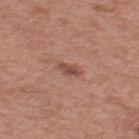The lesion was tiled from a total-body skin photograph and was not biopsied.
The tile uses white-light illumination.
A region of skin cropped from a whole-body photographic capture, roughly 15 mm wide.
The recorded lesion diameter is about 3 mm.
The total-body-photography lesion software estimated a shape eccentricity near 0.9 and two-axis asymmetry of about 0.3. And it measured a lesion color around L≈49 a*≈23 b*≈26 in CIELAB, about 10 CIELAB-L* units darker than the surrounding skin, and a normalized border contrast of about 7. It also reported a border-irregularity index near 3/10, internal color variation of about 2 on a 0–10 scale, and peripheral color asymmetry of about 0.5.
Located on the upper back.
A male subject aged 53 to 57.